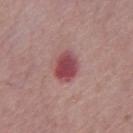The lesion was tiled from a total-body skin photograph and was not biopsied.
A male patient in their mid- to late 60s.
A lesion tile, about 15 mm wide, cut from a 3D total-body photograph.
Measured at roughly 4 mm in maximum diameter.
This is a white-light tile.
The lesion is on the chest.
The total-body-photography lesion software estimated a lesion area of about 8 mm² and two-axis asymmetry of about 0.1. And it measured an average lesion color of about L≈47 a*≈29 b*≈18 (CIELAB), a lesion–skin lightness drop of about 13, and a normalized lesion–skin contrast near 10. And it measured a border-irregularity index near 1/10, a within-lesion color-variation index near 4.5/10, and a peripheral color-asymmetry measure near 1.5.